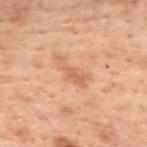  biopsy_status: not biopsied; imaged during a skin examination
  patient:
    sex: female
    age_approx: 55
  automated_metrics:
    area_mm2_approx: 5.0
    eccentricity: 0.9
    shape_asymmetry: 0.45
    color_variation_0_10: 1.0
    peripheral_color_asymmetry: 0.0
    nevus_likeness_0_100: 0
    lesion_detection_confidence_0_100: 100
  lesion_size:
    long_diameter_mm_approx: 4.0
  site: upper back
  image:
    source: total-body photography crop
    field_of_view_mm: 15